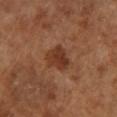notes: no biopsy performed (imaged during a skin exam); patient: female, roughly 65 years of age; size: ≈3.5 mm; body site: the left lower leg; tile lighting: cross-polarized illumination; acquisition: ~15 mm tile from a whole-body skin photo.